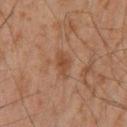Case summary:
• notes: catalogued during a skin exam; not biopsied
• acquisition: total-body-photography crop, ~15 mm field of view
• lesion diameter: ~3 mm (longest diameter)
• body site: the right lower leg
• patient: male, aged around 45
• image-analysis metrics: a border-irregularity rating of about 3.5/10, a color-variation rating of about 1.5/10, and radial color variation of about 0.5